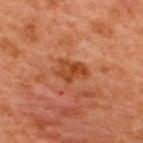  biopsy_status: not biopsied; imaged during a skin examination
  site: upper back
  image:
    source: total-body photography crop
    field_of_view_mm: 15
  lighting: cross-polarized
  patient:
    sex: male
    age_approx: 50
  lesion_size:
    long_diameter_mm_approx: 3.5